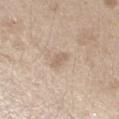Imaged during a routine full-body skin examination; the lesion was not biopsied and no histopathology is available. Imaged with white-light lighting. Automated tile analysis of the lesion measured about 8 CIELAB-L* units darker than the surrounding skin and a lesion-to-skin contrast of about 5 (normalized; higher = more distinct). The software also gave a border-irregularity rating of about 3/10, a color-variation rating of about 1/10, and radial color variation of about 0.5. The analysis additionally found an automated nevus-likeness rating near 0 out of 100. Cropped from a total-body skin-imaging series; the visible field is about 15 mm. Approximately 2.5 mm at its widest. The subject is a male about 70 years old. The lesion is located on the left forearm.